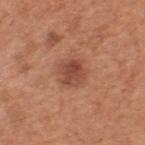No biopsy was performed on this lesion — it was imaged during a full skin examination and was not determined to be concerning.
Imaged with white-light lighting.
The lesion is located on the upper back.
A 15 mm close-up extracted from a 3D total-body photography capture.
The patient is a male aged approximately 55.